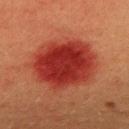Impression:
The lesion was tiled from a total-body skin photograph and was not biopsied.
Image and clinical context:
About 7 mm across. Cropped from a total-body skin-imaging series; the visible field is about 15 mm. On the mid back. A male subject in their 40s.